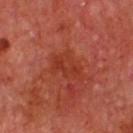Q: Was this lesion biopsied?
A: no biopsy performed (imaged during a skin exam)